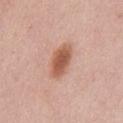Recorded during total-body skin imaging; not selected for excision or biopsy. Approximately 5 mm at its widest. Located on the abdomen. Imaged with white-light lighting. Cropped from a whole-body photographic skin survey; the tile spans about 15 mm. The patient is a male approximately 40 years of age.